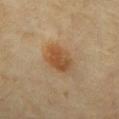Assessment:
No biopsy was performed on this lesion — it was imaged during a full skin examination and was not determined to be concerning.
Acquisition and patient details:
Imaged with cross-polarized lighting. Automated tile analysis of the lesion measured an area of roughly 9.5 mm², a shape eccentricity near 0.65, and two-axis asymmetry of about 0.15. And it measured a lesion color around L≈47 a*≈19 b*≈35 in CIELAB, roughly 9 lightness units darker than nearby skin, and a normalized border contrast of about 8. It also reported a nevus-likeness score of about 100/100 and a lesion-detection confidence of about 100/100. The patient is a male aged 58–62. The lesion is located on the mid back. Cropped from a whole-body photographic skin survey; the tile spans about 15 mm.